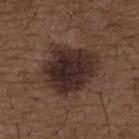Imaged during a routine full-body skin examination; the lesion was not biopsied and no histopathology is available. An algorithmic analysis of the crop reported a shape eccentricity near 0.65 and two-axis asymmetry of about 0.2. The software also gave a mean CIELAB color near L≈28 a*≈15 b*≈18, about 12 CIELAB-L* units darker than the surrounding skin, and a normalized lesion–skin contrast near 13. Approximately 6.5 mm at its widest. The patient is a male aged 48 to 52. A roughly 15 mm field-of-view crop from a total-body skin photograph. Captured under white-light illumination. Located on the upper back.